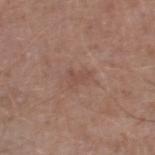{"patient": {"sex": "male", "age_approx": 65}, "site": "left lower leg", "image": {"source": "total-body photography crop", "field_of_view_mm": 15}}